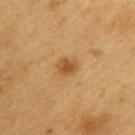{"biopsy_status": "not biopsied; imaged during a skin examination", "site": "upper back", "lesion_size": {"long_diameter_mm_approx": 2.5}, "image": {"source": "total-body photography crop", "field_of_view_mm": 15}, "lighting": "cross-polarized", "patient": {"sex": "male", "age_approx": 60}, "automated_metrics": {"cielab_L": 52, "cielab_a": 21, "cielab_b": 42, "vs_skin_darker_L": 10.0, "vs_skin_contrast_norm": 7.5, "nevus_likeness_0_100": 90, "lesion_detection_confidence_0_100": 100}}